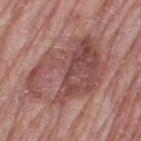follow-up = imaged on a skin check; not biopsied
image source = ~15 mm tile from a whole-body skin photo
anatomic site = the leg
subject = female, aged 68–72